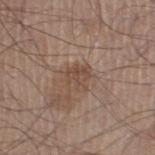Impression:
No biopsy was performed on this lesion — it was imaged during a full skin examination and was not determined to be concerning.
Acquisition and patient details:
The subject is a male in their mid-40s. This is a white-light tile. On the right lower leg. Measured at roughly 3 mm in maximum diameter. A roughly 15 mm field-of-view crop from a total-body skin photograph. The total-body-photography lesion software estimated a within-lesion color-variation index near 3.5/10 and radial color variation of about 1.5. And it measured a classifier nevus-likeness of about 0/100.